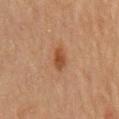{
  "biopsy_status": "not biopsied; imaged during a skin examination",
  "lesion_size": {
    "long_diameter_mm_approx": 3.0
  },
  "lighting": "cross-polarized",
  "patient": {
    "sex": "male",
    "age_approx": 70
  },
  "site": "mid back",
  "image": {
    "source": "total-body photography crop",
    "field_of_view_mm": 15
  },
  "automated_metrics": {
    "border_irregularity_0_10": 3.0,
    "color_variation_0_10": 2.5,
    "peripheral_color_asymmetry": 1.0,
    "nevus_likeness_0_100": 90,
    "lesion_detection_confidence_0_100": 100
  }
}